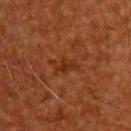Imaged during a routine full-body skin examination; the lesion was not biopsied and no histopathology is available. Imaged with cross-polarized lighting. A lesion tile, about 15 mm wide, cut from a 3D total-body photograph. Measured at roughly 3.5 mm in maximum diameter. The total-body-photography lesion software estimated an area of roughly 4.5 mm², an outline eccentricity of about 0.85 (0 = round, 1 = elongated), and a shape-asymmetry score of about 0.3 (0 = symmetric). A male patient aged around 60. From the upper back.